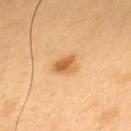Clinical summary: Longest diameter approximately 3 mm. This is a cross-polarized tile. The patient is a male about 50 years old. The total-body-photography lesion software estimated an average lesion color of about L≈62 a*≈23 b*≈43 (CIELAB), roughly 12 lightness units darker than nearby skin, and a normalized border contrast of about 8. The software also gave a nevus-likeness score of about 95/100 and a detector confidence of about 100 out of 100 that the crop contains a lesion. A 15 mm close-up tile from a total-body photography series done for melanoma screening. From the back.